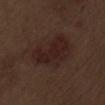Captured during whole-body skin photography for melanoma surveillance; the lesion was not biopsied.
Cropped from a total-body skin-imaging series; the visible field is about 15 mm.
The tile uses white-light illumination.
The recorded lesion diameter is about 7.5 mm.
The total-body-photography lesion software estimated a lesion color around L≈22 a*≈16 b*≈18 in CIELAB and a normalized lesion–skin contrast near 7.5.
A male subject, aged around 70.
The lesion is located on the left upper arm.